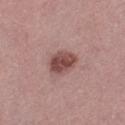Clinical impression: The lesion was photographed on a routine skin check and not biopsied; there is no pathology result. Context: The total-body-photography lesion software estimated a lesion area of about 8.5 mm² and an outline eccentricity of about 0.6 (0 = round, 1 = elongated). It also reported an average lesion color of about L≈48 a*≈22 b*≈22 (CIELAB), a lesion–skin lightness drop of about 13, and a normalized lesion–skin contrast near 9. It also reported a border-irregularity index near 1/10, internal color variation of about 4 on a 0–10 scale, and a peripheral color-asymmetry measure near 1.5. The software also gave a nevus-likeness score of about 85/100 and a lesion-detection confidence of about 100/100. Measured at roughly 3.5 mm in maximum diameter. A roughly 15 mm field-of-view crop from a total-body skin photograph. Captured under white-light illumination. Located on the leg. A female patient about 45 years old.